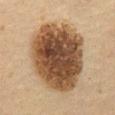Captured during whole-body skin photography for melanoma surveillance; the lesion was not biopsied.
An algorithmic analysis of the crop reported an average lesion color of about L≈39 a*≈15 b*≈28 (CIELAB), a lesion–skin lightness drop of about 17, and a normalized border contrast of about 13. And it measured a border-irregularity index near 1.5/10 and radial color variation of about 2. The software also gave a nevus-likeness score of about 90/100 and lesion-presence confidence of about 100/100.
A male subject, roughly 60 years of age.
The recorded lesion diameter is about 9.5 mm.
Located on the abdomen.
A 15 mm crop from a total-body photograph taken for skin-cancer surveillance.
This is a cross-polarized tile.